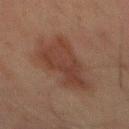Q: Was a biopsy performed?
A: imaged on a skin check; not biopsied
Q: What did automated image analysis measure?
A: a lesion area of about 20 mm² and two-axis asymmetry of about 0.35
Q: Where on the body is the lesion?
A: the front of the torso
Q: How large is the lesion?
A: about 7.5 mm
Q: How was the tile lit?
A: cross-polarized
Q: What kind of image is this?
A: ~15 mm tile from a whole-body skin photo
Q: What are the patient's age and sex?
A: male, in their mid- to late 60s